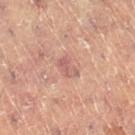<record>
  <biopsy_status>not biopsied; imaged during a skin examination</biopsy_status>
  <site>left leg</site>
  <patient>
    <sex>female</sex>
    <age_approx>80</age_approx>
  </patient>
  <image>
    <source>total-body photography crop</source>
    <field_of_view_mm>15</field_of_view_mm>
  </image>
</record>